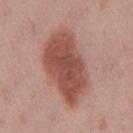Findings:
* workup · catalogued during a skin exam; not biopsied
* location · the mid back
* image · ~15 mm crop, total-body skin-cancer survey
* subject · male, approximately 45 years of age
* illumination · white-light